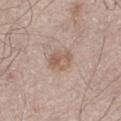Clinical impression:
Part of a total-body skin-imaging series; this lesion was reviewed on a skin check and was not flagged for biopsy.
Image and clinical context:
From the left thigh. Cropped from a total-body skin-imaging series; the visible field is about 15 mm. The tile uses white-light illumination. A male subject aged approximately 55.